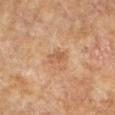Clinical impression:
The lesion was photographed on a routine skin check and not biopsied; there is no pathology result.
Context:
The lesion's longest dimension is about 2.5 mm. Automated image analysis of the tile measured an average lesion color of about L≈48 a*≈19 b*≈31 (CIELAB). It also reported border irregularity of about 2 on a 0–10 scale and peripheral color asymmetry of about 1. A female subject, aged approximately 80. Captured under cross-polarized illumination. Cropped from a whole-body photographic skin survey; the tile spans about 15 mm. On the arm.